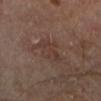notes: catalogued during a skin exam; not biopsied.